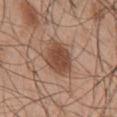The lesion was photographed on a routine skin check and not biopsied; there is no pathology result. The lesion is located on the front of the torso. The lesion's longest dimension is about 4.5 mm. Captured under white-light illumination. A lesion tile, about 15 mm wide, cut from a 3D total-body photograph. The lesion-visualizer software estimated a footprint of about 12 mm² and an outline eccentricity of about 0.6 (0 = round, 1 = elongated). The software also gave a border-irregularity index near 2/10 and a within-lesion color-variation index near 3/10. The analysis additionally found an automated nevus-likeness rating near 95 out of 100. A male patient in their mid- to late 40s.